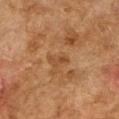Assessment:
The lesion was photographed on a routine skin check and not biopsied; there is no pathology result.
Context:
Captured under cross-polarized illumination. A 15 mm crop from a total-body photograph taken for skin-cancer surveillance. A female subject, in their 60s. About 2.5 mm across. The lesion is located on the right upper arm. An algorithmic analysis of the crop reported border irregularity of about 6 on a 0–10 scale.